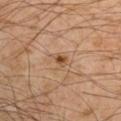Case summary:
* notes · catalogued during a skin exam; not biopsied
* patient · male, aged approximately 55
* image · total-body-photography crop, ~15 mm field of view
* anatomic site · the right upper arm
* lesion size · about 2 mm
* TBP lesion metrics · a shape eccentricity near 0.7 and a symmetry-axis asymmetry near 0.3; a lesion color around L≈48 a*≈20 b*≈34 in CIELAB and a lesion-to-skin contrast of about 8 (normalized; higher = more distinct)
* illumination · cross-polarized illumination